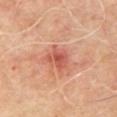Notes:
– notes — total-body-photography surveillance lesion; no biopsy
– acquisition — 15 mm crop, total-body photography
– anatomic site — the chest
– subject — male, approximately 50 years of age